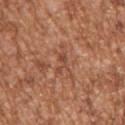{
  "biopsy_status": "not biopsied; imaged during a skin examination",
  "patient": {
    "sex": "male",
    "age_approx": 45
  },
  "site": "right upper arm",
  "lesion_size": {
    "long_diameter_mm_approx": 2.5
  },
  "automated_metrics": {
    "cielab_L": 47,
    "cielab_a": 24,
    "cielab_b": 31,
    "vs_skin_darker_L": 7.0
  },
  "image": {
    "source": "total-body photography crop",
    "field_of_view_mm": 15
  },
  "lighting": "white-light"
}